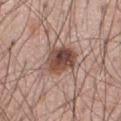workup: no biopsy performed (imaged during a skin exam) | image source: ~15 mm tile from a whole-body skin photo | tile lighting: white-light | lesion size: ~4.5 mm (longest diameter) | image-analysis metrics: an eccentricity of roughly 0.5; a border-irregularity index near 3/10, a within-lesion color-variation index near 8.5/10, and radial color variation of about 3; a nevus-likeness score of about 90/100 and a lesion-detection confidence of about 100/100 | body site: the chest | subject: male, aged 63 to 67.